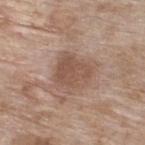Notes:
– biopsy status · total-body-photography surveillance lesion; no biopsy
– image source · total-body-photography crop, ~15 mm field of view
– body site · the upper back
– illumination · white-light illumination
– subject · female, about 75 years old